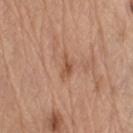The lesion was tiled from a total-body skin photograph and was not biopsied. Imaged with white-light lighting. From the left upper arm. A 15 mm close-up extracted from a 3D total-body photography capture. Longest diameter approximately 2.5 mm. A male subject, roughly 70 years of age.